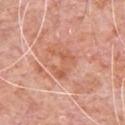<tbp_lesion>
  <biopsy_status>not biopsied; imaged during a skin examination</biopsy_status>
  <patient>
    <sex>male</sex>
    <age_approx>80</age_approx>
  </patient>
  <lesion_size>
    <long_diameter_mm_approx>4.0</long_diameter_mm_approx>
  </lesion_size>
  <image>
    <source>total-body photography crop</source>
    <field_of_view_mm>15</field_of_view_mm>
  </image>
  <site>chest</site>
  <lighting>white-light</lighting>
  <automated_metrics>
    <area_mm2_approx>7.5</area_mm2_approx>
    <eccentricity>0.7</eccentricity>
    <border_irregularity_0_10>9.0</border_irregularity_0_10>
    <color_variation_0_10>2.5</color_variation_0_10>
  </automated_metrics>
</tbp_lesion>